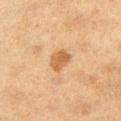Q: What is the imaging modality?
A: ~15 mm tile from a whole-body skin photo
Q: What is the lesion's diameter?
A: ~3 mm (longest diameter)
Q: Patient demographics?
A: female, about 40 years old
Q: Where on the body is the lesion?
A: the left thigh
Q: What did automated image analysis measure?
A: an average lesion color of about L≈52 a*≈19 b*≈36 (CIELAB), roughly 10 lightness units darker than nearby skin, and a lesion-to-skin contrast of about 7.5 (normalized; higher = more distinct); a border-irregularity rating of about 1.5/10, a within-lesion color-variation index near 2/10, and radial color variation of about 1